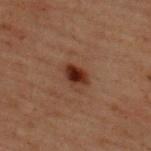Case summary:
* notes: imaged on a skin check; not biopsied
* image: ~15 mm crop, total-body skin-cancer survey
* anatomic site: the back
* diameter: ≈2.5 mm
* patient: male, aged 58 to 62
* tile lighting: cross-polarized